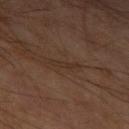Recorded during total-body skin imaging; not selected for excision or biopsy. Captured under cross-polarized illumination. The lesion is on the left upper arm. The subject is a male approximately 65 years of age. A 15 mm close-up tile from a total-body photography series done for melanoma screening. About 3.5 mm across.